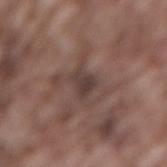anatomic site: the lower back | image: total-body-photography crop, ~15 mm field of view | subject: male, aged approximately 75.